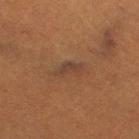follow-up: imaged on a skin check; not biopsied
image: ~15 mm tile from a whole-body skin photo
anatomic site: the right thigh
tile lighting: cross-polarized illumination
patient: female, about 55 years old